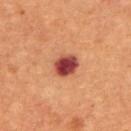Background: A male patient, roughly 65 years of age. Cropped from a total-body skin-imaging series; the visible field is about 15 mm. The total-body-photography lesion software estimated a mean CIELAB color near L≈42 a*≈31 b*≈27, a lesion–skin lightness drop of about 17, and a normalized lesion–skin contrast near 14. The software also gave a border-irregularity index near 1.5/10, internal color variation of about 5.5 on a 0–10 scale, and peripheral color asymmetry of about 1.5. The lesion is on the chest.